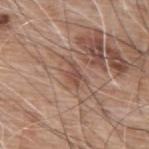Located on the upper back. Cropped from a whole-body photographic skin survey; the tile spans about 15 mm. Approximately 3 mm at its widest. The patient is a male aged approximately 60.